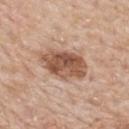subject: male, roughly 65 years of age | location: the mid back | image: ~15 mm tile from a whole-body skin photo | illumination: white-light.